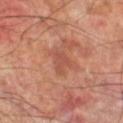Impression:
Captured during whole-body skin photography for melanoma surveillance; the lesion was not biopsied.
Clinical summary:
A lesion tile, about 15 mm wide, cut from a 3D total-body photograph. About 3 mm across. The tile uses cross-polarized illumination. Located on the right lower leg. A male subject roughly 70 years of age.